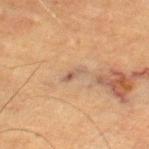The lesion was tiled from a total-body skin photograph and was not biopsied. A 15 mm close-up extracted from a 3D total-body photography capture. Located on the right thigh. Automated tile analysis of the lesion measured a shape eccentricity near 0.9 and a symmetry-axis asymmetry near 0.25. The analysis additionally found a border-irregularity rating of about 3/10, internal color variation of about 2 on a 0–10 scale, and peripheral color asymmetry of about 0.5. And it measured a nevus-likeness score of about 0/100 and lesion-presence confidence of about 55/100. A male subject, aged approximately 85 or older. Measured at roughly 3 mm in maximum diameter.